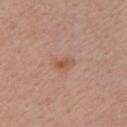Clinical impression: The lesion was tiled from a total-body skin photograph and was not biopsied. Clinical summary: Imaged with white-light lighting. The lesion is located on the left upper arm. About 2.5 mm across. The subject is a female aged approximately 55. Cropped from a total-body skin-imaging series; the visible field is about 15 mm.